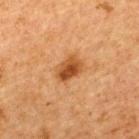biopsy status — total-body-photography surveillance lesion; no biopsy | lesion diameter — ≈3.5 mm | image source — ~15 mm tile from a whole-body skin photo | body site — the back | patient — male, aged 63–67 | automated metrics — lesion-presence confidence of about 100/100 | illumination — cross-polarized illumination.